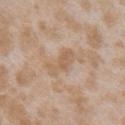Imaged during a routine full-body skin examination; the lesion was not biopsied and no histopathology is available. This image is a 15 mm lesion crop taken from a total-body photograph. Automated image analysis of the tile measured a lesion area of about 5 mm², a shape eccentricity near 0.9, and a symmetry-axis asymmetry near 0.35. The analysis additionally found internal color variation of about 1.5 on a 0–10 scale and radial color variation of about 0.5. It also reported a nevus-likeness score of about 0/100 and lesion-presence confidence of about 100/100. A female patient aged around 25. About 3.5 mm across. The lesion is located on the right upper arm. This is a white-light tile.